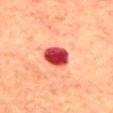{
  "biopsy_status": "not biopsied; imaged during a skin examination",
  "image": {
    "source": "total-body photography crop",
    "field_of_view_mm": 15
  },
  "lesion_size": {
    "long_diameter_mm_approx": 3.5
  },
  "site": "mid back",
  "lighting": "cross-polarized",
  "patient": {
    "sex": "female",
    "age_approx": 65
  }
}